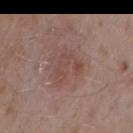follow-up=total-body-photography surveillance lesion; no biopsy
location=the right upper arm
illumination=white-light
image=~15 mm tile from a whole-body skin photo
TBP lesion metrics=a shape eccentricity near 0.65 and two-axis asymmetry of about 0.55; a border-irregularity rating of about 7.5/10, a color-variation rating of about 3/10, and peripheral color asymmetry of about 1; a nevus-likeness score of about 0/100 and a lesion-detection confidence of about 100/100
subject=male, aged approximately 55